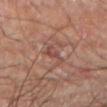Q: Was this lesion biopsied?
A: no biopsy performed (imaged during a skin exam)
Q: Who is the patient?
A: male, approximately 60 years of age
Q: What is the imaging modality?
A: total-body-photography crop, ~15 mm field of view
Q: Lesion size?
A: about 3.5 mm
Q: How was the tile lit?
A: cross-polarized illumination
Q: Where on the body is the lesion?
A: the left forearm